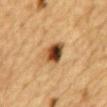The lesion was photographed on a routine skin check and not biopsied; there is no pathology result.
The tile uses cross-polarized illumination.
Located on the mid back.
A region of skin cropped from a whole-body photographic capture, roughly 15 mm wide.
Measured at roughly 4 mm in maximum diameter.
A male patient approximately 85 years of age or older.
Automated tile analysis of the lesion measured an average lesion color of about L≈41 a*≈18 b*≈33 (CIELAB), a lesion–skin lightness drop of about 17, and a lesion-to-skin contrast of about 13 (normalized; higher = more distinct). The analysis additionally found a color-variation rating of about 10/10. And it measured a nevus-likeness score of about 100/100 and lesion-presence confidence of about 100/100.